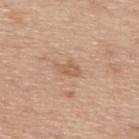The tile uses white-light illumination. A male patient, roughly 75 years of age. The lesion is on the upper back. A region of skin cropped from a whole-body photographic capture, roughly 15 mm wide. The recorded lesion diameter is about 3 mm.